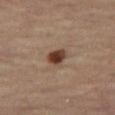From the left leg.
Measured at roughly 2.5 mm in maximum diameter.
This is a cross-polarized tile.
A male subject aged 68–72.
A 15 mm crop from a total-body photograph taken for skin-cancer surveillance.